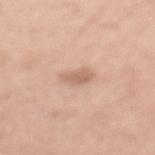A female patient aged 48–52.
From the back.
The tile uses white-light illumination.
A region of skin cropped from a whole-body photographic capture, roughly 15 mm wide.